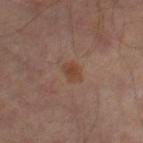- follow-up: imaged on a skin check; not biopsied
- patient: male, roughly 65 years of age
- imaging modality: ~15 mm crop, total-body skin-cancer survey
- size: about 2.5 mm
- site: the right thigh